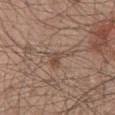Clinical impression: The lesion was tiled from a total-body skin photograph and was not biopsied. Acquisition and patient details: Captured under white-light illumination. An algorithmic analysis of the crop reported a lesion area of about 5 mm² and a symmetry-axis asymmetry near 0.6. The analysis additionally found a mean CIELAB color near L≈48 a*≈16 b*≈26, about 8 CIELAB-L* units darker than the surrounding skin, and a normalized border contrast of about 6. And it measured lesion-presence confidence of about 90/100. Longest diameter approximately 4 mm. A male subject, roughly 50 years of age. On the mid back. Cropped from a whole-body photographic skin survey; the tile spans about 15 mm.